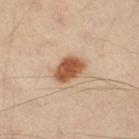{
  "biopsy_status": "not biopsied; imaged during a skin examination",
  "site": "right thigh",
  "image": {
    "source": "total-body photography crop",
    "field_of_view_mm": 15
  },
  "patient": {
    "age_approx": 55
  }
}